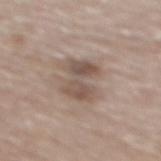{"biopsy_status": "not biopsied; imaged during a skin examination", "lighting": "white-light", "lesion_size": {"long_diameter_mm_approx": 4.5}, "automated_metrics": {"border_irregularity_0_10": 5.5, "color_variation_0_10": 4.5, "peripheral_color_asymmetry": 1.5, "nevus_likeness_0_100": 15, "lesion_detection_confidence_0_100": 100}, "patient": {"sex": "male", "age_approx": 75}, "site": "mid back", "image": {"source": "total-body photography crop", "field_of_view_mm": 15}}